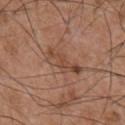follow-up: total-body-photography surveillance lesion; no biopsy
location: the chest
TBP lesion metrics: an average lesion color of about L≈46 a*≈21 b*≈29 (CIELAB), about 8 CIELAB-L* units darker than the surrounding skin, and a normalized lesion–skin contrast near 6.5; a border-irregularity index near 5/10 and internal color variation of about 4 on a 0–10 scale
lesion diameter: ~5 mm (longest diameter)
acquisition: 15 mm crop, total-body photography
tile lighting: white-light
patient: male, aged 53–57